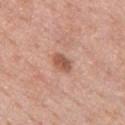Impression:
Captured during whole-body skin photography for melanoma surveillance; the lesion was not biopsied.
Clinical summary:
The patient is a male aged approximately 60. A close-up tile cropped from a whole-body skin photograph, about 15 mm across. Automated tile analysis of the lesion measured a lesion area of about 4.5 mm² and a shape eccentricity near 0.75. And it measured about 12 CIELAB-L* units darker than the surrounding skin and a normalized lesion–skin contrast near 8. And it measured a border-irregularity index near 1.5/10. From the chest. Measured at roughly 3 mm in maximum diameter.